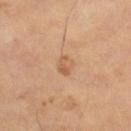Clinical impression:
Imaged during a routine full-body skin examination; the lesion was not biopsied and no histopathology is available.
Image and clinical context:
A 15 mm close-up extracted from a 3D total-body photography capture. A male subject in their mid-60s. The lesion is on the left leg.